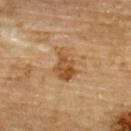Findings:
* follow-up: catalogued during a skin exam; not biopsied
* patient: male, roughly 85 years of age or older
* body site: the upper back
* illumination: cross-polarized
* size: ≈4 mm
* image source: ~15 mm tile from a whole-body skin photo
* automated lesion analysis: a border-irregularity rating of about 4/10 and a within-lesion color-variation index near 5/10